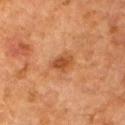Captured during whole-body skin photography for melanoma surveillance; the lesion was not biopsied.
The patient is a male aged approximately 60.
The lesion is on the left arm.
Imaged with cross-polarized lighting.
Measured at roughly 3 mm in maximum diameter.
A 15 mm close-up tile from a total-body photography series done for melanoma screening.